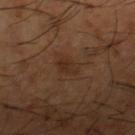Assessment:
The lesion was tiled from a total-body skin photograph and was not biopsied.
Acquisition and patient details:
On the leg. Captured under cross-polarized illumination. The subject is a male aged around 65. A lesion tile, about 15 mm wide, cut from a 3D total-body photograph. The lesion-visualizer software estimated a footprint of about 4 mm², a shape eccentricity near 0.85, and two-axis asymmetry of about 0.3. The software also gave an average lesion color of about L≈29 a*≈18 b*≈27 (CIELAB), about 6 CIELAB-L* units darker than the surrounding skin, and a normalized lesion–skin contrast near 6. It also reported an automated nevus-likeness rating near 0 out of 100 and a lesion-detection confidence of about 100/100. The lesion's longest dimension is about 3 mm.